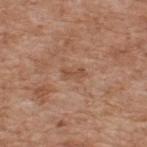notes=no biopsy performed (imaged during a skin exam) | site=the upper back | tile lighting=white-light | image source=~15 mm crop, total-body skin-cancer survey | subject=male, aged 58–62 | size=~2.5 mm (longest diameter).